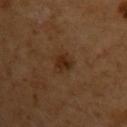This lesion was catalogued during total-body skin photography and was not selected for biopsy.
The tile uses cross-polarized illumination.
About 3 mm across.
A lesion tile, about 15 mm wide, cut from a 3D total-body photograph.
On the left upper arm.
A female subject in their mid- to late 50s.